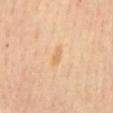Assessment:
Imaged during a routine full-body skin examination; the lesion was not biopsied and no histopathology is available.
Clinical summary:
This is a cross-polarized tile. An algorithmic analysis of the crop reported a border-irregularity rating of about 2/10, a within-lesion color-variation index near 0/10, and peripheral color asymmetry of about 0. It also reported an automated nevus-likeness rating near 0 out of 100. Measured at roughly 2.5 mm in maximum diameter. A close-up tile cropped from a whole-body skin photograph, about 15 mm across. Located on the mid back. The subject is a female in their mid- to late 60s.